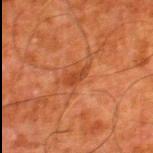Captured during whole-body skin photography for melanoma surveillance; the lesion was not biopsied. This is a cross-polarized tile. A male subject roughly 80 years of age. The lesion-visualizer software estimated a footprint of about 3 mm², a shape eccentricity near 0.85, and two-axis asymmetry of about 0.4. The software also gave a nevus-likeness score of about 0/100 and a detector confidence of about 100 out of 100 that the crop contains a lesion. A close-up tile cropped from a whole-body skin photograph, about 15 mm across. From the leg.